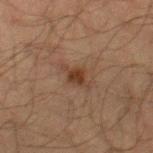image — 15 mm crop, total-body photography
size — ~3.5 mm (longest diameter)
location — the right thigh
tile lighting — cross-polarized illumination
patient — male, approximately 65 years of age